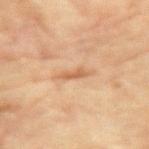Clinical impression:
Part of a total-body skin-imaging series; this lesion was reviewed on a skin check and was not flagged for biopsy.
Acquisition and patient details:
On the arm. A female patient, aged 78–82. Automated image analysis of the tile measured a shape eccentricity near 0.95 and a shape-asymmetry score of about 0.35 (0 = symmetric). The software also gave border irregularity of about 4 on a 0–10 scale, a color-variation rating of about 0/10, and radial color variation of about 0. The software also gave an automated nevus-likeness rating near 0 out of 100 and lesion-presence confidence of about 100/100. The lesion's longest dimension is about 3 mm. A region of skin cropped from a whole-body photographic capture, roughly 15 mm wide. This is a cross-polarized tile.